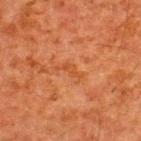size=≈3 mm
image-analysis metrics=a classifier nevus-likeness of about 0/100 and lesion-presence confidence of about 100/100
subject=male, aged around 60
lighting=cross-polarized
site=the upper back
acquisition=total-body-photography crop, ~15 mm field of view